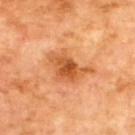Findings:
* workup: catalogued during a skin exam; not biopsied
* image: ~15 mm crop, total-body skin-cancer survey
* diameter: ~5 mm (longest diameter)
* location: the upper back
* patient: male, in their 70s